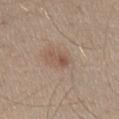biopsy_status: not biopsied; imaged during a skin examination
lighting: white-light
lesion_size:
  long_diameter_mm_approx: 2.5
patient:
  sex: male
  age_approx: 30
site: right upper arm
image:
  source: total-body photography crop
  field_of_view_mm: 15